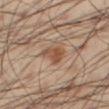site=the leg
acquisition=~15 mm crop, total-body skin-cancer survey
subject=male, about 55 years old
automated lesion analysis=a mean CIELAB color near L≈51 a*≈20 b*≈31, roughly 10 lightness units darker than nearby skin, and a normalized border contrast of about 7.5; a lesion-detection confidence of about 100/100
diameter=≈3 mm